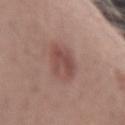Context:
Located on the left forearm. The patient is a male aged 28–32. A 15 mm close-up extracted from a 3D total-body photography capture.